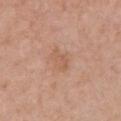Assessment:
The lesion was tiled from a total-body skin photograph and was not biopsied.